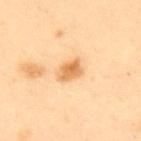workup — total-body-photography surveillance lesion; no biopsy
image source — total-body-photography crop, ~15 mm field of view
patient — female, aged around 40
body site — the upper back